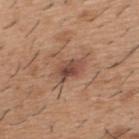Imaged during a routine full-body skin examination; the lesion was not biopsied and no histopathology is available.
The recorded lesion diameter is about 3.5 mm.
A lesion tile, about 15 mm wide, cut from a 3D total-body photograph.
The tile uses white-light illumination.
On the upper back.
A male subject in their 40s.